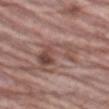The lesion was tiled from a total-body skin photograph and was not biopsied. The subject is a male roughly 70 years of age. A lesion tile, about 15 mm wide, cut from a 3D total-body photograph. This is a white-light tile. The lesion's longest dimension is about 5.5 mm. Located on the left thigh.